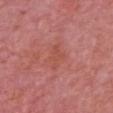biopsy_status: not biopsied; imaged during a skin examination
patient:
  sex: male
  age_approx: 85+
site: front of the torso
image:
  source: total-body photography crop
  field_of_view_mm: 15